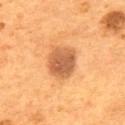The lesion was photographed on a routine skin check and not biopsied; there is no pathology result. Measured at roughly 4 mm in maximum diameter. A male patient roughly 55 years of age. The lesion is on the back. Automated image analysis of the tile measured a lesion area of about 12 mm² and two-axis asymmetry of about 0.15. The software also gave a mean CIELAB color near L≈55 a*≈24 b*≈38. It also reported an automated nevus-likeness rating near 80 out of 100 and a detector confidence of about 100 out of 100 that the crop contains a lesion. A lesion tile, about 15 mm wide, cut from a 3D total-body photograph.